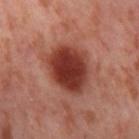follow-up — total-body-photography surveillance lesion; no biopsy
image source — total-body-photography crop, ~15 mm field of view
patient — female, aged around 55
anatomic site — the right thigh